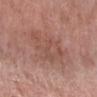Part of a total-body skin-imaging series; this lesion was reviewed on a skin check and was not flagged for biopsy. About 8 mm across. Automated tile analysis of the lesion measured roughly 8 lightness units darker than nearby skin and a normalized lesion–skin contrast near 5.5. The patient is a female in their mid- to late 60s. This is a white-light tile. A 15 mm crop from a total-body photograph taken for skin-cancer surveillance. The lesion is on the right forearm.